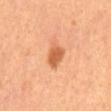Q: Was a biopsy performed?
A: imaged on a skin check; not biopsied
Q: What is the lesion's diameter?
A: ≈3.5 mm
Q: What did automated image analysis measure?
A: a footprint of about 5.5 mm², an eccentricity of roughly 0.75, and a symmetry-axis asymmetry near 0.3; an average lesion color of about L≈61 a*≈29 b*≈42 (CIELAB), roughly 13 lightness units darker than nearby skin, and a normalized border contrast of about 8
Q: Illumination type?
A: cross-polarized illumination
Q: Who is the patient?
A: female
Q: What kind of image is this?
A: ~15 mm tile from a whole-body skin photo
Q: Where on the body is the lesion?
A: the front of the torso